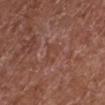Recorded during total-body skin imaging; not selected for excision or biopsy.
A region of skin cropped from a whole-body photographic capture, roughly 15 mm wide.
Measured at roughly 3 mm in maximum diameter.
A male patient aged 73–77.
Automated tile analysis of the lesion measured an outline eccentricity of about 0.65 (0 = round, 1 = elongated) and a shape-asymmetry score of about 0.55 (0 = symmetric). The analysis additionally found an automated nevus-likeness rating near 0 out of 100 and a detector confidence of about 95 out of 100 that the crop contains a lesion.
From the left lower leg.